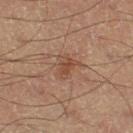Impression: The lesion was photographed on a routine skin check and not biopsied; there is no pathology result. Background: A 15 mm close-up extracted from a 3D total-body photography capture. Automated image analysis of the tile measured an automated nevus-likeness rating near 20 out of 100. Measured at roughly 3 mm in maximum diameter. The tile uses cross-polarized illumination. A male patient aged 58 to 62. Located on the right thigh.